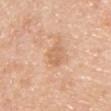  biopsy_status: not biopsied; imaged during a skin examination
  patient:
    sex: male
    age_approx: 35
  site: front of the torso
  image:
    source: total-body photography crop
    field_of_view_mm: 15